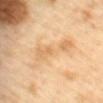- subject — female, aged around 60
- tile lighting — cross-polarized illumination
- site — the mid back
- acquisition — 15 mm crop, total-body photography
- diameter — about 6.5 mm